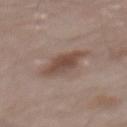biopsy_status: not biopsied; imaged during a skin examination
lighting: white-light
site: mid back
lesion_size:
  long_diameter_mm_approx: 5.0
patient:
  sex: male
  age_approx: 55
automated_metrics:
  area_mm2_approx: 10.0
  shape_asymmetry: 0.25
  cielab_L: 47
  cielab_a: 17
  cielab_b: 24
  vs_skin_darker_L: 10.0
  vs_skin_contrast_norm: 8.0
  nevus_likeness_0_100: 40
image:
  source: total-body photography crop
  field_of_view_mm: 15